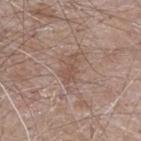<tbp_lesion>
<biopsy_status>not biopsied; imaged during a skin examination</biopsy_status>
<image>
  <source>total-body photography crop</source>
  <field_of_view_mm>15</field_of_view_mm>
</image>
<patient>
  <sex>male</sex>
  <age_approx>65</age_approx>
</patient>
<site>chest</site>
<lesion_size>
  <long_diameter_mm_approx>3.5</long_diameter_mm_approx>
</lesion_size>
</tbp_lesion>